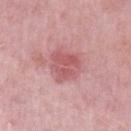This lesion was catalogued during total-body skin photography and was not selected for biopsy. This image is a 15 mm lesion crop taken from a total-body photograph. Located on the left upper arm. This is a white-light tile. A female subject, aged 43–47.